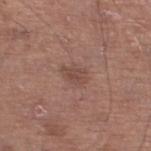<tbp_lesion>
  <biopsy_status>not biopsied; imaged during a skin examination</biopsy_status>
  <automated_metrics>
    <area_mm2_approx>5.0</area_mm2_approx>
    <eccentricity>0.7</eccentricity>
    <shape_asymmetry>0.25</shape_asymmetry>
    <nevus_likeness_0_100>15</nevus_likeness_0_100>
    <lesion_detection_confidence_0_100>100</lesion_detection_confidence_0_100>
  </automated_metrics>
  <image>
    <source>total-body photography crop</source>
    <field_of_view_mm>15</field_of_view_mm>
  </image>
  <patient>
    <sex>male</sex>
    <age_approx>65</age_approx>
  </patient>
  <lighting>white-light</lighting>
  <site>left lower leg</site>
  <lesion_size>
    <long_diameter_mm_approx>3.0</long_diameter_mm_approx>
  </lesion_size>
</tbp_lesion>